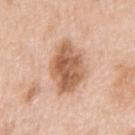follow-up — catalogued during a skin exam; not biopsied | acquisition — total-body-photography crop, ~15 mm field of view | diameter — ~6 mm (longest diameter) | lighting — white-light illumination | location — the right upper arm | subject — female, roughly 45 years of age | automated lesion analysis — a lesion area of about 18 mm², an eccentricity of roughly 0.75, and a shape-asymmetry score of about 0.15 (0 = symmetric); peripheral color asymmetry of about 2.